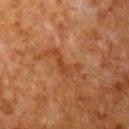Captured during whole-body skin photography for melanoma surveillance; the lesion was not biopsied.
A 15 mm close-up extracted from a 3D total-body photography capture.
From the chest.
A male subject, in their 80s.
Captured under cross-polarized illumination.